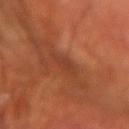Q: Was a biopsy performed?
A: catalogued during a skin exam; not biopsied